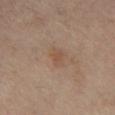Impression:
Captured during whole-body skin photography for melanoma surveillance; the lesion was not biopsied.
Context:
A male subject, aged approximately 60. The lesion's longest dimension is about 2.5 mm. The lesion is on the abdomen. This is a cross-polarized tile. Cropped from a total-body skin-imaging series; the visible field is about 15 mm.